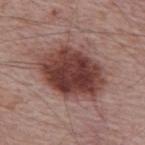notes: catalogued during a skin exam; not biopsied | lesion size: ~7.5 mm (longest diameter) | patient: male, about 65 years old | tile lighting: white-light illumination | location: the upper back | imaging modality: total-body-photography crop, ~15 mm field of view | image-analysis metrics: an area of roughly 31 mm², an eccentricity of roughly 0.7, and a shape-asymmetry score of about 0.15 (0 = symmetric); a mean CIELAB color near L≈40 a*≈23 b*≈22, about 16 CIELAB-L* units darker than the surrounding skin, and a lesion-to-skin contrast of about 12 (normalized; higher = more distinct); border irregularity of about 2 on a 0–10 scale, a color-variation rating of about 6/10, and a peripheral color-asymmetry measure near 2.